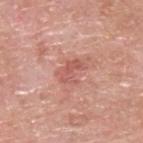Recorded during total-body skin imaging; not selected for excision or biopsy.
Measured at roughly 3 mm in maximum diameter.
The subject is a male in their mid- to late 60s.
Located on the upper back.
The lesion-visualizer software estimated a lesion–skin lightness drop of about 9. It also reported a classifier nevus-likeness of about 0/100 and a lesion-detection confidence of about 100/100.
Imaged with white-light lighting.
This image is a 15 mm lesion crop taken from a total-body photograph.